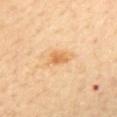| key | value |
|---|---|
| follow-up | total-body-photography surveillance lesion; no biopsy |
| lesion size | ~3.5 mm (longest diameter) |
| site | the back |
| automated metrics | a footprint of about 5 mm², an outline eccentricity of about 0.85 (0 = round, 1 = elongated), and two-axis asymmetry of about 0.25; a border-irregularity index near 2/10 and a within-lesion color-variation index near 3.5/10; an automated nevus-likeness rating near 30 out of 100 |
| image | ~15 mm crop, total-body skin-cancer survey |
| subject | female, about 70 years old |